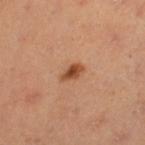<lesion>
  <biopsy_status>not biopsied; imaged during a skin examination</biopsy_status>
  <lesion_size>
    <long_diameter_mm_approx>2.5</long_diameter_mm_approx>
  </lesion_size>
  <patient>
    <sex>female</sex>
    <age_approx>35</age_approx>
  </patient>
  <site>left thigh</site>
  <image>
    <source>total-body photography crop</source>
    <field_of_view_mm>15</field_of_view_mm>
  </image>
</lesion>